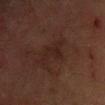Q: Was this lesion biopsied?
A: catalogued during a skin exam; not biopsied
Q: Lesion size?
A: about 4 mm
Q: Illumination type?
A: cross-polarized
Q: What is the imaging modality?
A: total-body-photography crop, ~15 mm field of view
Q: What is the anatomic site?
A: the right forearm
Q: Patient demographics?
A: male, approximately 60 years of age